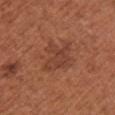follow-up = total-body-photography surveillance lesion; no biopsy | body site = the chest | patient = female, aged 63 to 67 | imaging modality = ~15 mm tile from a whole-body skin photo | lesion size = ~4.5 mm (longest diameter) | lighting = white-light.